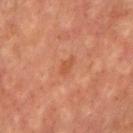Q: What lighting was used for the tile?
A: cross-polarized illumination
Q: Who is the patient?
A: male, about 70 years old
Q: How large is the lesion?
A: about 2.5 mm
Q: What kind of image is this?
A: ~15 mm tile from a whole-body skin photo
Q: Automated lesion metrics?
A: an area of roughly 3 mm², a shape eccentricity near 0.85, and two-axis asymmetry of about 0.4; an automated nevus-likeness rating near 0 out of 100 and lesion-presence confidence of about 100/100
Q: Where on the body is the lesion?
A: the front of the torso